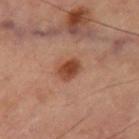{
  "biopsy_status": "not biopsied; imaged during a skin examination",
  "automated_metrics": {
    "eccentricity": 0.7,
    "shape_asymmetry": 0.15
  },
  "site": "right thigh",
  "lighting": "cross-polarized",
  "image": {
    "source": "total-body photography crop",
    "field_of_view_mm": 15
  },
  "lesion_size": {
    "long_diameter_mm_approx": 3.0
  }
}